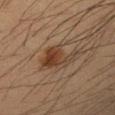Context: A 15 mm close-up tile from a total-body photography series done for melanoma screening. A female patient, aged 33–37. From the arm. Measured at roughly 5 mm in maximum diameter. The lesion-visualizer software estimated an area of roughly 10 mm², an eccentricity of roughly 0.7, and a shape-asymmetry score of about 0.4 (0 = symmetric). The analysis additionally found a border-irregularity rating of about 5/10 and radial color variation of about 2. The analysis additionally found a nevus-likeness score of about 100/100 and a lesion-detection confidence of about 100/100.